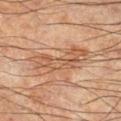• follow-up · no biopsy performed (imaged during a skin exam)
• patient · male, roughly 65 years of age
• anatomic site · the right lower leg
• automated lesion analysis · a lesion area of about 10 mm² and a symmetry-axis asymmetry near 0.6; border irregularity of about 9 on a 0–10 scale and radial color variation of about 1; a classifier nevus-likeness of about 0/100 and lesion-presence confidence of about 75/100
• size · ~7 mm (longest diameter)
• imaging modality · ~15 mm crop, total-body skin-cancer survey